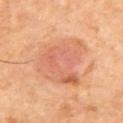Case summary:
– tile lighting: cross-polarized illumination
– automated metrics: a color-variation rating of about 5.5/10 and peripheral color asymmetry of about 1.5
– image source: total-body-photography crop, ~15 mm field of view
– anatomic site: the upper back
– patient: male, approximately 60 years of age